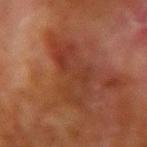follow-up: total-body-photography surveillance lesion; no biopsy | patient: male, aged approximately 70 | size: about 6 mm | body site: the right upper arm | image source: total-body-photography crop, ~15 mm field of view | illumination: cross-polarized illumination.